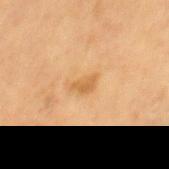<record>
<biopsy_status>not biopsied; imaged during a skin examination</biopsy_status>
<lesion_size>
  <long_diameter_mm_approx>3.0</long_diameter_mm_approx>
</lesion_size>
<image>
  <source>total-body photography crop</source>
  <field_of_view_mm>15</field_of_view_mm>
</image>
<lighting>cross-polarized</lighting>
<patient>
  <sex>male</sex>
  <age_approx>60</age_approx>
</patient>
</record>